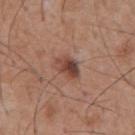{"biopsy_status": "not biopsied; imaged during a skin examination", "patient": {"sex": "male", "age_approx": 55}, "lesion_size": {"long_diameter_mm_approx": 3.5}, "image": {"source": "total-body photography crop", "field_of_view_mm": 15}, "lighting": "white-light", "site": "chest"}